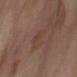biopsy status = total-body-photography surveillance lesion; no biopsy | lesion diameter = about 3.5 mm | image = ~15 mm crop, total-body skin-cancer survey | site = the abdomen | patient = female, about 55 years old | tile lighting = cross-polarized.